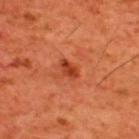Impression: This lesion was catalogued during total-body skin photography and was not selected for biopsy. Clinical summary: Captured under cross-polarized illumination. Approximately 2.5 mm at its widest. A male subject about 60 years old. A roughly 15 mm field-of-view crop from a total-body skin photograph. The lesion is located on the upper back.